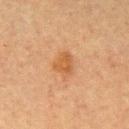biopsy status: no biopsy performed (imaged during a skin exam); lighting: cross-polarized illumination; size: ~3 mm (longest diameter); patient: female, roughly 55 years of age; anatomic site: the right upper arm; acquisition: ~15 mm crop, total-body skin-cancer survey.